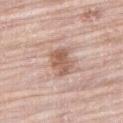This lesion was catalogued during total-body skin photography and was not selected for biopsy. Measured at roughly 3.5 mm in maximum diameter. Automated tile analysis of the lesion measured a lesion area of about 7.5 mm², an eccentricity of roughly 0.65, and a symmetry-axis asymmetry near 0.25. It also reported a border-irregularity index near 2.5/10, a color-variation rating of about 3.5/10, and radial color variation of about 1.5. And it measured a nevus-likeness score of about 35/100. On the right thigh. Captured under white-light illumination. A male subject, aged around 80. A roughly 15 mm field-of-view crop from a total-body skin photograph.